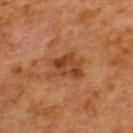The lesion was photographed on a routine skin check and not biopsied; there is no pathology result.
A male subject, about 65 years old.
The lesion is on the upper back.
Measured at roughly 5 mm in maximum diameter.
A roughly 15 mm field-of-view crop from a total-body skin photograph.